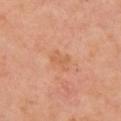Notes:
- biopsy status · total-body-photography surveillance lesion; no biopsy
- size · ~2.5 mm (longest diameter)
- subject · female, in their mid-50s
- lighting · cross-polarized illumination
- image source · ~15 mm crop, total-body skin-cancer survey
- location · the right upper arm
- TBP lesion metrics · a border-irregularity index near 2.5/10, a color-variation rating of about 1.5/10, and peripheral color asymmetry of about 0.5